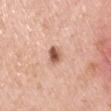Assessment:
This lesion was catalogued during total-body skin photography and was not selected for biopsy.
Acquisition and patient details:
The patient is a male in their 40s. The lesion is on the arm. A roughly 15 mm field-of-view crop from a total-body skin photograph.